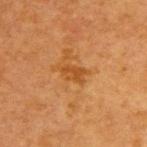Q: Was this lesion biopsied?
A: total-body-photography surveillance lesion; no biopsy
Q: Automated lesion metrics?
A: an area of roughly 4 mm²; border irregularity of about 2.5 on a 0–10 scale, internal color variation of about 1.5 on a 0–10 scale, and peripheral color asymmetry of about 0.5; an automated nevus-likeness rating near 5 out of 100 and lesion-presence confidence of about 100/100
Q: Patient demographics?
A: female, aged around 40
Q: How large is the lesion?
A: ~3 mm (longest diameter)
Q: How was the tile lit?
A: cross-polarized illumination
Q: What is the imaging modality?
A: total-body-photography crop, ~15 mm field of view
Q: What is the anatomic site?
A: the upper back